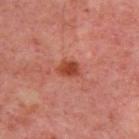Notes:
• notes — imaged on a skin check; not biopsied
• lighting — cross-polarized illumination
• site — the back
• patient — aged around 55
• lesion diameter — about 3 mm
• acquisition — ~15 mm tile from a whole-body skin photo